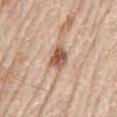Assessment:
Part of a total-body skin-imaging series; this lesion was reviewed on a skin check and was not flagged for biopsy.
Background:
About 3.5 mm across. An algorithmic analysis of the crop reported a mean CIELAB color near L≈57 a*≈21 b*≈30, roughly 15 lightness units darker than nearby skin, and a lesion-to-skin contrast of about 9.5 (normalized; higher = more distinct). And it measured a border-irregularity rating of about 2.5/10, a within-lesion color-variation index near 5.5/10, and peripheral color asymmetry of about 1.5. And it measured an automated nevus-likeness rating near 100 out of 100 and a detector confidence of about 100 out of 100 that the crop contains a lesion. The subject is a male approximately 80 years of age. This is a white-light tile. Cropped from a whole-body photographic skin survey; the tile spans about 15 mm. The lesion is located on the mid back.